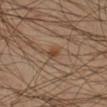Assessment: Recorded during total-body skin imaging; not selected for excision or biopsy. Image and clinical context: The lesion-visualizer software estimated an average lesion color of about L≈42 a*≈17 b*≈30 (CIELAB), roughly 8 lightness units darker than nearby skin, and a normalized border contrast of about 7.5. And it measured border irregularity of about 1.5 on a 0–10 scale and a peripheral color-asymmetry measure near 1. Located on the right lower leg. The patient is a male about 45 years old. Cropped from a whole-body photographic skin survey; the tile spans about 15 mm.